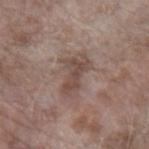• follow-up · total-body-photography surveillance lesion; no biopsy
• image · 15 mm crop, total-body photography
• anatomic site · the left forearm
• tile lighting · white-light illumination
• lesion size · about 5 mm
• patient · female, about 75 years old